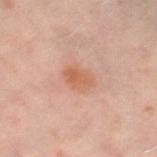Assessment:
Imaged during a routine full-body skin examination; the lesion was not biopsied and no histopathology is available.
Acquisition and patient details:
The lesion's longest dimension is about 3.5 mm. A 15 mm crop from a total-body photograph taken for skin-cancer surveillance. A female subject roughly 50 years of age. On the left thigh.